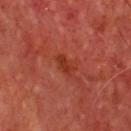This lesion was catalogued during total-body skin photography and was not selected for biopsy. A 15 mm close-up tile from a total-body photography series done for melanoma screening. The lesion's longest dimension is about 3 mm. The tile uses cross-polarized illumination. From the front of the torso. A male patient aged approximately 65.